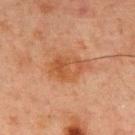The lesion was tiled from a total-body skin photograph and was not biopsied. A 15 mm close-up tile from a total-body photography series done for melanoma screening. The tile uses cross-polarized illumination. About 4.5 mm across. The subject is a male aged around 65. On the chest. Automated tile analysis of the lesion measured an average lesion color of about L≈42 a*≈21 b*≈32 (CIELAB) and a normalized lesion–skin contrast near 6.5.